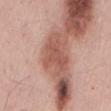No biopsy was performed on this lesion — it was imaged during a full skin examination and was not determined to be concerning.
A male patient aged around 55.
From the mid back.
Cropped from a whole-body photographic skin survey; the tile spans about 15 mm.